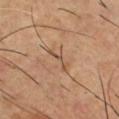workup=imaged on a skin check; not biopsied | subject=male, aged around 55 | size=about 4.5 mm | image source=15 mm crop, total-body photography | site=the chest | automated lesion analysis=a mean CIELAB color near L≈53 a*≈17 b*≈31, roughly 6 lightness units darker than nearby skin, and a normalized lesion–skin contrast near 4.5 | tile lighting=cross-polarized illumination.